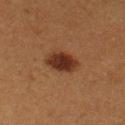Impression:
Recorded during total-body skin imaging; not selected for excision or biopsy.
Context:
The lesion is located on the leg. A close-up tile cropped from a whole-body skin photograph, about 15 mm across. A female subject, roughly 40 years of age.